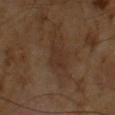workup: total-body-photography surveillance lesion; no biopsy
image-analysis metrics: an area of roughly 5 mm², a shape eccentricity near 0.8, and two-axis asymmetry of about 0.4; a lesion–skin lightness drop of about 5 and a lesion-to-skin contrast of about 5 (normalized; higher = more distinct)
size: about 3 mm
imaging modality: 15 mm crop, total-body photography
subject: male, about 65 years old
tile lighting: cross-polarized